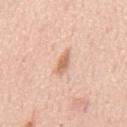notes: no biopsy performed (imaged during a skin exam) | tile lighting: white-light illumination | image source: total-body-photography crop, ~15 mm field of view | automated lesion analysis: an area of roughly 3.5 mm², an eccentricity of roughly 0.9, and a symmetry-axis asymmetry near 0.3; about 12 CIELAB-L* units darker than the surrounding skin and a lesion-to-skin contrast of about 7.5 (normalized; higher = more distinct) | subject: male, aged around 65 | body site: the mid back.